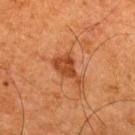Clinical impression:
No biopsy was performed on this lesion — it was imaged during a full skin examination and was not determined to be concerning.
Clinical summary:
The subject is a male approximately 65 years of age. The lesion is located on the upper back. Cropped from a total-body skin-imaging series; the visible field is about 15 mm.